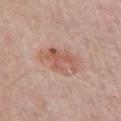{"biopsy_status": "not biopsied; imaged during a skin examination", "lesion_size": {"long_diameter_mm_approx": 5.0}, "lighting": "white-light", "patient": {"sex": "male", "age_approx": 65}, "image": {"source": "total-body photography crop", "field_of_view_mm": 15}, "site": "front of the torso", "automated_metrics": {"area_mm2_approx": 13.0, "eccentricity": 0.75, "cielab_L": 58, "cielab_a": 22, "cielab_b": 27, "vs_skin_darker_L": 9.0, "vs_skin_contrast_norm": 6.5, "nevus_likeness_0_100": 85, "lesion_detection_confidence_0_100": 100}}